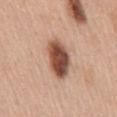Q: Patient demographics?
A: female, aged 58–62
Q: What did automated image analysis measure?
A: an area of roughly 12 mm²; a lesion color around L≈49 a*≈22 b*≈28 in CIELAB, roughly 20 lightness units darker than nearby skin, and a normalized lesion–skin contrast near 13; a border-irregularity index near 1.5/10, internal color variation of about 7 on a 0–10 scale, and a peripheral color-asymmetry measure near 2.5; a lesion-detection confidence of about 100/100
Q: Where on the body is the lesion?
A: the mid back
Q: How was this image acquired?
A: ~15 mm tile from a whole-body skin photo